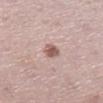Assessment: Part of a total-body skin-imaging series; this lesion was reviewed on a skin check and was not flagged for biopsy. Image and clinical context: A female patient, in their 40s. Approximately 2.5 mm at its widest. The lesion-visualizer software estimated a footprint of about 3.5 mm², a shape eccentricity near 0.7, and a symmetry-axis asymmetry near 0.2. And it measured a lesion color around L≈57 a*≈20 b*≈23 in CIELAB, roughly 13 lightness units darker than nearby skin, and a lesion-to-skin contrast of about 8.5 (normalized; higher = more distinct). And it measured a nevus-likeness score of about 95/100 and a lesion-detection confidence of about 100/100. This is a white-light tile. The lesion is on the left lower leg. Cropped from a total-body skin-imaging series; the visible field is about 15 mm.